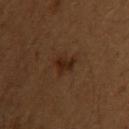Findings:
- workup — imaged on a skin check; not biopsied